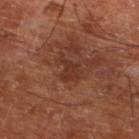  biopsy_status: not biopsied; imaged during a skin examination
  site: left lower leg
  lesion_size:
    long_diameter_mm_approx: 3.0
  image:
    source: total-body photography crop
    field_of_view_mm: 15
  patient:
    age_approx: 65
  lighting: cross-polarized